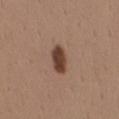<case>
  <biopsy_status>not biopsied; imaged during a skin examination</biopsy_status>
  <site>mid back</site>
  <image>
    <source>total-body photography crop</source>
    <field_of_view_mm>15</field_of_view_mm>
  </image>
  <lighting>white-light</lighting>
  <patient>
    <sex>male</sex>
    <age_approx>40</age_approx>
  </patient>
  <lesion_size>
    <long_diameter_mm_approx>3.5</long_diameter_mm_approx>
  </lesion_size>
</case>